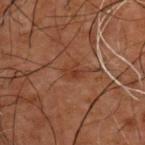This lesion was catalogued during total-body skin photography and was not selected for biopsy.
The subject is a male in their 50s.
A roughly 15 mm field-of-view crop from a total-body skin photograph.
Imaged with cross-polarized lighting.
From the upper back.
The recorded lesion diameter is about 2.5 mm.